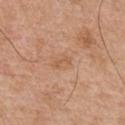Impression: The lesion was tiled from a total-body skin photograph and was not biopsied. Acquisition and patient details: A male subject aged approximately 55. The lesion is on the front of the torso. A close-up tile cropped from a whole-body skin photograph, about 15 mm across. This is a white-light tile. Longest diameter approximately 2.5 mm. The lesion-visualizer software estimated a footprint of about 2.5 mm², an outline eccentricity of about 0.9 (0 = round, 1 = elongated), and a shape-asymmetry score of about 0.45 (0 = symmetric). And it measured a lesion color around L≈57 a*≈21 b*≈35 in CIELAB, a lesion–skin lightness drop of about 6, and a normalized lesion–skin contrast near 5. It also reported a within-lesion color-variation index near 0/10 and peripheral color asymmetry of about 0. The analysis additionally found a nevus-likeness score of about 0/100 and lesion-presence confidence of about 100/100.